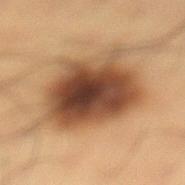notes = no biopsy performed (imaged during a skin exam) | imaging modality = total-body-photography crop, ~15 mm field of view | tile lighting = cross-polarized | automated lesion analysis = a lesion–skin lightness drop of about 17 and a normalized lesion–skin contrast near 13; an automated nevus-likeness rating near 70 out of 100 and lesion-presence confidence of about 100/100 | location = the left lower leg | diameter = about 8 mm | patient = male, about 35 years old.